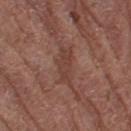Context: From the leg. This is a white-light tile. An algorithmic analysis of the crop reported a lesion color around L≈41 a*≈21 b*≈24 in CIELAB, roughly 7 lightness units darker than nearby skin, and a lesion-to-skin contrast of about 5.5 (normalized; higher = more distinct). A female patient aged 78 to 82. A lesion tile, about 15 mm wide, cut from a 3D total-body photograph.